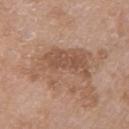Notes:
* image source · ~15 mm tile from a whole-body skin photo
* patient · female, roughly 70 years of age
* lesion diameter · ≈7.5 mm
* location · the left upper arm